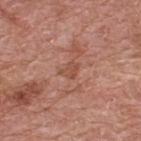Captured during whole-body skin photography for melanoma surveillance; the lesion was not biopsied.